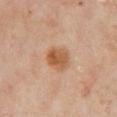Q: Is there a histopathology result?
A: total-body-photography surveillance lesion; no biopsy
Q: Automated lesion metrics?
A: a lesion area of about 7.5 mm², an outline eccentricity of about 0.55 (0 = round, 1 = elongated), and a symmetry-axis asymmetry near 0.15; a mean CIELAB color near L≈56 a*≈22 b*≈36 and a lesion–skin lightness drop of about 10; a nevus-likeness score of about 95/100 and a lesion-detection confidence of about 100/100
Q: What is the anatomic site?
A: the chest
Q: How large is the lesion?
A: ~3.5 mm (longest diameter)
Q: Illumination type?
A: cross-polarized
Q: How was this image acquired?
A: total-body-photography crop, ~15 mm field of view
Q: Who is the patient?
A: female, aged approximately 60